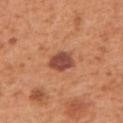Assessment: The lesion was photographed on a routine skin check and not biopsied; there is no pathology result. Clinical summary: From the left upper arm. A male subject, about 55 years old. Cropped from a whole-body photographic skin survey; the tile spans about 15 mm. This is a white-light tile.